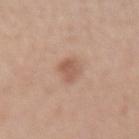Recorded during total-body skin imaging; not selected for excision or biopsy. The tile uses white-light illumination. Approximately 3 mm at its widest. On the right forearm. This image is a 15 mm lesion crop taken from a total-body photograph. The lesion-visualizer software estimated a border-irregularity rating of about 3/10, a within-lesion color-variation index near 2.5/10, and radial color variation of about 1. A female subject about 40 years old.